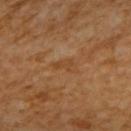| feature | finding |
|---|---|
| notes | catalogued during a skin exam; not biopsied |
| image source | 15 mm crop, total-body photography |
| TBP lesion metrics | an eccentricity of roughly 0.85 and two-axis asymmetry of about 0.45; a lesion color around L≈43 a*≈20 b*≈36 in CIELAB and a lesion-to-skin contrast of about 4.5 (normalized; higher = more distinct) |
| site | the upper back |
| illumination | cross-polarized illumination |
| subject | female, aged approximately 65 |
| lesion diameter | ≈3.5 mm |